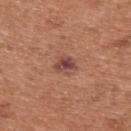Notes:
– notes: imaged on a skin check; not biopsied
– TBP lesion metrics: a lesion color around L≈46 a*≈24 b*≈26 in CIELAB, a lesion–skin lightness drop of about 10, and a normalized lesion–skin contrast near 8; a classifier nevus-likeness of about 45/100 and a lesion-detection confidence of about 100/100
– imaging modality: 15 mm crop, total-body photography
– lesion size: about 3 mm
– location: the upper back
– patient: male, roughly 55 years of age
– lighting: white-light illumination